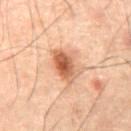Notes:
- patient: male, aged 58–62
- automated lesion analysis: an area of roughly 9 mm², a shape eccentricity near 0.75, and a shape-asymmetry score of about 0.2 (0 = symmetric); a mean CIELAB color near L≈46 a*≈20 b*≈28 and a lesion–skin lightness drop of about 12; a border-irregularity index near 2.5/10, a color-variation rating of about 6.5/10, and a peripheral color-asymmetry measure near 2
- anatomic site: the abdomen
- lesion diameter: ≈4 mm
- image source: ~15 mm crop, total-body skin-cancer survey